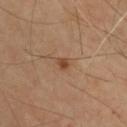<lesion>
  <biopsy_status>not biopsied; imaged during a skin examination</biopsy_status>
  <lesion_size>
    <long_diameter_mm_approx>1.5</long_diameter_mm_approx>
  </lesion_size>
  <image>
    <source>total-body photography crop</source>
    <field_of_view_mm>15</field_of_view_mm>
  </image>
  <lighting>cross-polarized</lighting>
  <patient>
    <sex>male</sex>
    <age_approx>65</age_approx>
  </patient>
  <site>back</site>
  <automated_metrics>
    <area_mm2_approx>2.0</area_mm2_approx>
    <eccentricity>0.45</eccentricity>
    <shape_asymmetry>0.3</shape_asymmetry>
    <border_irregularity_0_10>2.5</border_irregularity_0_10>
    <peripheral_color_asymmetry>0.5</peripheral_color_asymmetry>
  </automated_metrics>
</lesion>